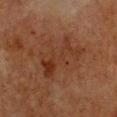follow-up: catalogued during a skin exam; not biopsied | imaging modality: total-body-photography crop, ~15 mm field of view | illumination: cross-polarized | subject: female, in their mid- to late 50s | body site: the chest | image-analysis metrics: an average lesion color of about L≈28 a*≈19 b*≈27 (CIELAB), roughly 5 lightness units darker than nearby skin, and a lesion-to-skin contrast of about 6 (normalized; higher = more distinct); border irregularity of about 8 on a 0–10 scale, a color-variation rating of about 3.5/10, and peripheral color asymmetry of about 1.